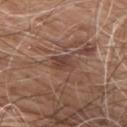This lesion was catalogued during total-body skin photography and was not selected for biopsy. The subject is a male aged 58–62. The lesion is located on the right upper arm. A 15 mm close-up extracted from a 3D total-body photography capture. The tile uses white-light illumination.